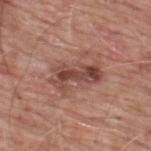Q: Was this lesion biopsied?
A: imaged on a skin check; not biopsied
Q: What did automated image analysis measure?
A: a nevus-likeness score of about 5/100 and a detector confidence of about 100 out of 100 that the crop contains a lesion
Q: What is the imaging modality?
A: ~15 mm tile from a whole-body skin photo
Q: What is the anatomic site?
A: the upper back
Q: How was the tile lit?
A: white-light illumination
Q: What are the patient's age and sex?
A: male, aged 58–62
Q: How large is the lesion?
A: ~5.5 mm (longest diameter)